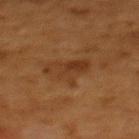Impression: Captured during whole-body skin photography for melanoma surveillance; the lesion was not biopsied. Clinical summary: The patient is a female aged 48 to 52. From the upper back. Measured at roughly 4.5 mm in maximum diameter. A region of skin cropped from a whole-body photographic capture, roughly 15 mm wide.